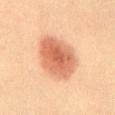<tbp_lesion>
<image>
  <source>total-body photography crop</source>
  <field_of_view_mm>15</field_of_view_mm>
</image>
<patient>
  <sex>male</sex>
  <age_approx>60</age_approx>
</patient>
<site>lower back</site>
</tbp_lesion>